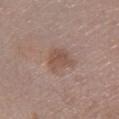Captured during whole-body skin photography for melanoma surveillance; the lesion was not biopsied.
Imaged with white-light lighting.
A 15 mm crop from a total-body photograph taken for skin-cancer surveillance.
The lesion is on the left lower leg.
The patient is a male aged around 55.